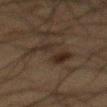  lesion_size:
    long_diameter_mm_approx: 5.0
  patient:
    sex: male
    age_approx: 60
  image:
    source: total-body photography crop
    field_of_view_mm: 15
  automated_metrics:
    area_mm2_approx: 10.0
    eccentricity: 0.85
    shape_asymmetry: 0.4
    vs_skin_darker_L: 6.0
    vs_skin_contrast_norm: 7.5
    border_irregularity_0_10: 7.5
    peripheral_color_asymmetry: 1.5
  lighting: cross-polarized
  site: mid back